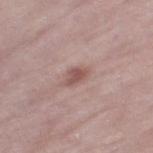{
  "biopsy_status": "not biopsied; imaged during a skin examination",
  "lesion_size": {
    "long_diameter_mm_approx": 3.0
  },
  "lighting": "white-light",
  "site": "left thigh",
  "image": {
    "source": "total-body photography crop",
    "field_of_view_mm": 15
  },
  "patient": {
    "sex": "female",
    "age_approx": 70
  }
}